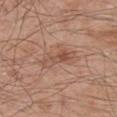The lesion was tiled from a total-body skin photograph and was not biopsied. Longest diameter approximately 4 mm. An algorithmic analysis of the crop reported an area of roughly 5.5 mm² and two-axis asymmetry of about 0.3. It also reported an average lesion color of about L≈51 a*≈21 b*≈28 (CIELAB), a lesion–skin lightness drop of about 8, and a normalized lesion–skin contrast near 6. It also reported border irregularity of about 4 on a 0–10 scale, internal color variation of about 3.5 on a 0–10 scale, and a peripheral color-asymmetry measure near 0.5. And it measured an automated nevus-likeness rating near 0 out of 100 and a detector confidence of about 100 out of 100 that the crop contains a lesion. A region of skin cropped from a whole-body photographic capture, roughly 15 mm wide. From the right upper arm. The tile uses white-light illumination. A male subject, approximately 45 years of age.